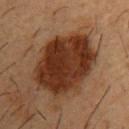Clinical impression:
The lesion was tiled from a total-body skin photograph and was not biopsied.